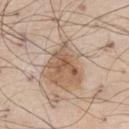Imaged during a routine full-body skin examination; the lesion was not biopsied and no histopathology is available. The subject is a male aged approximately 70. The tile uses white-light illumination. On the right thigh. This image is a 15 mm lesion crop taken from a total-body photograph.